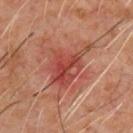Findings:
– tile lighting: cross-polarized
– subject: male, aged approximately 60
– location: the chest
– lesion diameter: about 6 mm
– image source: total-body-photography crop, ~15 mm field of view
– automated lesion analysis: a lesion color around L≈43 a*≈30 b*≈28 in CIELAB, roughly 9 lightness units darker than nearby skin, and a normalized lesion–skin contrast near 7.5; a border-irregularity index near 5/10, internal color variation of about 6.5 on a 0–10 scale, and radial color variation of about 2; an automated nevus-likeness rating near 0 out of 100 and a lesion-detection confidence of about 100/100